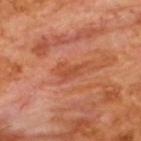{
  "biopsy_status": "not biopsied; imaged during a skin examination",
  "automated_metrics": {
    "area_mm2_approx": 4.0,
    "eccentricity": 0.9,
    "shape_asymmetry": 0.45,
    "border_irregularity_0_10": 5.5,
    "color_variation_0_10": 1.5,
    "peripheral_color_asymmetry": 0.5,
    "nevus_likeness_0_100": 0,
    "lesion_detection_confidence_0_100": 100
  },
  "lesion_size": {
    "long_diameter_mm_approx": 3.5
  },
  "patient": {
    "sex": "male",
    "age_approx": 65
  },
  "image": {
    "source": "total-body photography crop",
    "field_of_view_mm": 15
  },
  "lighting": "cross-polarized"
}